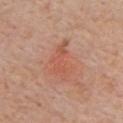Recorded during total-body skin imaging; not selected for excision or biopsy.
The tile uses white-light illumination.
Automated tile analysis of the lesion measured an area of roughly 8.5 mm² and an outline eccentricity of about 0.85 (0 = round, 1 = elongated).
A close-up tile cropped from a whole-body skin photograph, about 15 mm across.
A male patient roughly 60 years of age.
About 5 mm across.
Located on the front of the torso.